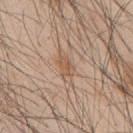Q: Was a biopsy performed?
A: no biopsy performed (imaged during a skin exam)
Q: What is the anatomic site?
A: the mid back
Q: What kind of image is this?
A: ~15 mm crop, total-body skin-cancer survey
Q: Patient demographics?
A: male, about 45 years old